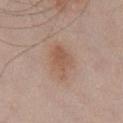Notes:
– notes — catalogued during a skin exam; not biopsied
– lesion size — about 4.5 mm
– tile lighting — white-light
– imaging modality — ~15 mm crop, total-body skin-cancer survey
– body site — the chest
– patient — male, aged approximately 55